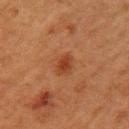– workup — total-body-photography surveillance lesion; no biopsy
– illumination — cross-polarized illumination
– image source — ~15 mm tile from a whole-body skin photo
– lesion diameter — ~3 mm (longest diameter)
– anatomic site — the arm
– patient — female, aged around 50